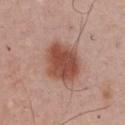* biopsy status · catalogued during a skin exam; not biopsied
* image source · ~15 mm tile from a whole-body skin photo
* patient · male, approximately 60 years of age
* lighting · white-light illumination
* location · the chest